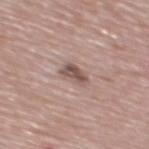| feature | finding |
|---|---|
| biopsy status | total-body-photography surveillance lesion; no biopsy |
| body site | the mid back |
| automated lesion analysis | a mean CIELAB color near L≈52 a*≈17 b*≈21, roughly 12 lightness units darker than nearby skin, and a normalized border contrast of about 8.5; a border-irregularity index near 3.5/10 and a peripheral color-asymmetry measure near 1.5 |
| size | about 2.5 mm |
| image | ~15 mm crop, total-body skin-cancer survey |
| subject | male, about 70 years old |